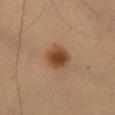biopsy_status: not biopsied; imaged during a skin examination
lesion_size:
  long_diameter_mm_approx: 3.5
patient:
  sex: male
  age_approx: 55
site: front of the torso
image:
  source: total-body photography crop
  field_of_view_mm: 15